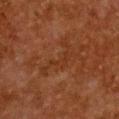Notes:
– workup — no biopsy performed (imaged during a skin exam)
– imaging modality — total-body-photography crop, ~15 mm field of view
– patient — female, approximately 50 years of age
– diameter — ~5.5 mm (longest diameter)
– site — the chest